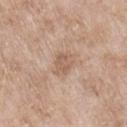workup: no biopsy performed (imaged during a skin exam)